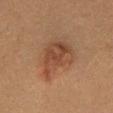No biopsy was performed on this lesion — it was imaged during a full skin examination and was not determined to be concerning. The lesion is located on the chest. About 6.5 mm across. The subject is a male about 50 years old. A lesion tile, about 15 mm wide, cut from a 3D total-body photograph.